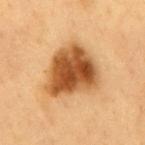| field | value |
|---|---|
| image source | ~15 mm crop, total-body skin-cancer survey |
| TBP lesion metrics | a border-irregularity rating of about 3/10, a color-variation rating of about 7.5/10, and a peripheral color-asymmetry measure near 2.5; a classifier nevus-likeness of about 100/100 and a detector confidence of about 100 out of 100 that the crop contains a lesion |
| patient | male, aged approximately 60 |
| body site | the mid back |
| diameter | about 6 mm |